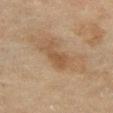follow-up: catalogued during a skin exam; not biopsied
subject: female, aged 58 to 62
body site: the left thigh
imaging modality: total-body-photography crop, ~15 mm field of view
automated lesion analysis: an outline eccentricity of about 0.85 (0 = round, 1 = elongated) and two-axis asymmetry of about 0.4; an average lesion color of about L≈47 a*≈16 b*≈31 (CIELAB), roughly 7 lightness units darker than nearby skin, and a normalized border contrast of about 6; border irregularity of about 4.5 on a 0–10 scale; a nevus-likeness score of about 0/100 and lesion-presence confidence of about 100/100
lighting: cross-polarized
lesion diameter: about 3.5 mm